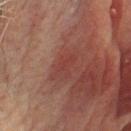| field | value |
|---|---|
| follow-up | no biopsy performed (imaged during a skin exam) |
| TBP lesion metrics | a lesion area of about 4.5 mm², an eccentricity of roughly 0.8, and two-axis asymmetry of about 0.35; a lesion color around L≈33 a*≈23 b*≈22 in CIELAB, a lesion–skin lightness drop of about 5, and a lesion-to-skin contrast of about 4.5 (normalized; higher = more distinct) |
| size | about 3 mm |
| subject | male, in their mid-70s |
| lighting | cross-polarized illumination |
| location | the head or neck |
| image | ~15 mm crop, total-body skin-cancer survey |